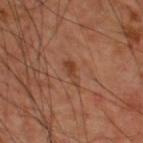<case>
<biopsy_status>not biopsied; imaged during a skin examination</biopsy_status>
<site>upper back</site>
<lighting>cross-polarized</lighting>
<patient>
  <sex>male</sex>
  <age_approx>60</age_approx>
</patient>
<image>
  <source>total-body photography crop</source>
  <field_of_view_mm>15</field_of_view_mm>
</image>
</case>